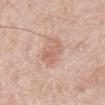This lesion was catalogued during total-body skin photography and was not selected for biopsy.
Captured under white-light illumination.
The subject is a male in their 80s.
The lesion's longest dimension is about 4 mm.
The lesion is located on the mid back.
A lesion tile, about 15 mm wide, cut from a 3D total-body photograph.